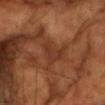Case summary:
• follow-up: catalogued during a skin exam; not biopsied
• illumination: cross-polarized illumination
• patient: male, aged 58–62
• location: the head or neck
• automated lesion analysis: a mean CIELAB color near L≈34 a*≈24 b*≈30, roughly 6 lightness units darker than nearby skin, and a normalized lesion–skin contrast near 5.5; a border-irregularity index near 5.5/10, internal color variation of about 2 on a 0–10 scale, and peripheral color asymmetry of about 0.5; a nevus-likeness score of about 0/100 and a lesion-detection confidence of about 85/100
• imaging modality: total-body-photography crop, ~15 mm field of view